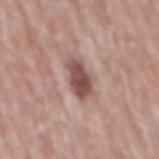Assessment:
This lesion was catalogued during total-body skin photography and was not selected for biopsy.
Clinical summary:
Longest diameter approximately 4 mm. Automated tile analysis of the lesion measured a lesion area of about 7 mm². The software also gave border irregularity of about 2 on a 0–10 scale and peripheral color asymmetry of about 1. On the mid back. Cropped from a total-body skin-imaging series; the visible field is about 15 mm. The patient is a male roughly 70 years of age.